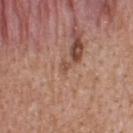A region of skin cropped from a whole-body photographic capture, roughly 15 mm wide. Automated tile analysis of the lesion measured an outline eccentricity of about 0.65 (0 = round, 1 = elongated) and a symmetry-axis asymmetry near 0.5. The software also gave a mean CIELAB color near L≈49 a*≈22 b*≈29, about 8 CIELAB-L* units darker than the surrounding skin, and a normalized border contrast of about 6. It also reported an automated nevus-likeness rating near 0 out of 100 and lesion-presence confidence of about 95/100. About 1 mm across. The lesion is located on the upper back. A male patient approximately 40 years of age.